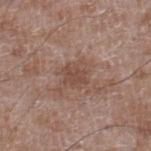biopsy status: imaged on a skin check; not biopsied
lighting: white-light
image source: ~15 mm crop, total-body skin-cancer survey
subject: male, about 60 years old
lesion size: ~2.5 mm (longest diameter)
site: the right lower leg